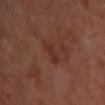A roughly 15 mm field-of-view crop from a total-body skin photograph. The lesion is located on the chest. A female patient aged approximately 50. Imaged with cross-polarized lighting.